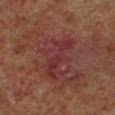No biopsy was performed on this lesion — it was imaged during a full skin examination and was not determined to be concerning. Longest diameter approximately 6 mm. A close-up tile cropped from a whole-body skin photograph, about 15 mm across. Automated image analysis of the tile measured an average lesion color of about L≈31 a*≈25 b*≈20 (CIELAB), roughly 5 lightness units darker than nearby skin, and a normalized border contrast of about 6. The analysis additionally found internal color variation of about 3 on a 0–10 scale and peripheral color asymmetry of about 1. The software also gave a classifier nevus-likeness of about 0/100 and a lesion-detection confidence of about 95/100. The tile uses cross-polarized illumination. On the left lower leg. The subject is a male aged approximately 60.